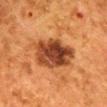{
  "biopsy_status": "not biopsied; imaged during a skin examination",
  "image": {
    "source": "total-body photography crop",
    "field_of_view_mm": 15
  },
  "site": "mid back",
  "patient": {
    "sex": "female",
    "age_approx": 50
  }
}